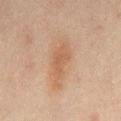On the abdomen. A region of skin cropped from a whole-body photographic capture, roughly 15 mm wide. The lesion's longest dimension is about 4.5 mm. Imaged with cross-polarized lighting. A male patient aged approximately 45.